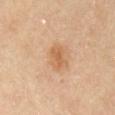biopsy status = no biopsy performed (imaged during a skin exam)
acquisition = ~15 mm crop, total-body skin-cancer survey
site = the chest
lighting = cross-polarized illumination
image-analysis metrics = roughly 8 lightness units darker than nearby skin and a normalized lesion–skin contrast near 6.5; a classifier nevus-likeness of about 60/100 and lesion-presence confidence of about 100/100
subject = male, in their mid- to late 80s or older
lesion diameter = about 3 mm